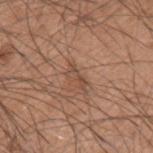Recorded during total-body skin imaging; not selected for excision or biopsy. A close-up tile cropped from a whole-body skin photograph, about 15 mm across. The lesion is on the left upper arm. This is a white-light tile. A male patient, approximately 60 years of age.